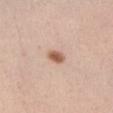No biopsy was performed on this lesion — it was imaged during a full skin examination and was not determined to be concerning.
On the left thigh.
This is a white-light tile.
Automated image analysis of the tile measured a footprint of about 3.5 mm², a shape eccentricity near 0.8, and two-axis asymmetry of about 0.25. It also reported a border-irregularity index near 2/10, a color-variation rating of about 2.5/10, and peripheral color asymmetry of about 0.5.
A female patient, about 35 years old.
Cropped from a total-body skin-imaging series; the visible field is about 15 mm.
The recorded lesion diameter is about 2.5 mm.